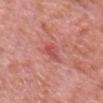Imaged during a routine full-body skin examination; the lesion was not biopsied and no histopathology is available. The lesion is on the head or neck. The subject is a male aged around 80. A 15 mm crop from a total-body photograph taken for skin-cancer surveillance. This is a white-light tile. About 2.5 mm across.